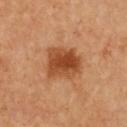The lesion was tiled from a total-body skin photograph and was not biopsied. On the chest. Cropped from a whole-body photographic skin survey; the tile spans about 15 mm. This is a cross-polarized tile. The lesion's longest dimension is about 4.5 mm. A female patient, in their 60s.